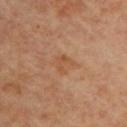biopsy status = no biopsy performed (imaged during a skin exam); image = ~15 mm tile from a whole-body skin photo; size = about 2.5 mm; subject = female, in their 60s; location = the upper back.